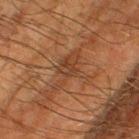Part of a total-body skin-imaging series; this lesion was reviewed on a skin check and was not flagged for biopsy. Located on the leg. A male subject, roughly 65 years of age. The tile uses cross-polarized illumination. A lesion tile, about 15 mm wide, cut from a 3D total-body photograph.